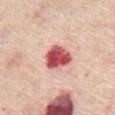Cropped from a whole-body photographic skin survey; the tile spans about 15 mm.
On the front of the torso.
A male patient approximately 75 years of age.
The lesion-visualizer software estimated an area of roughly 9.5 mm² and an outline eccentricity of about 0.25 (0 = round, 1 = elongated). It also reported an average lesion color of about L≈56 a*≈36 b*≈26 (CIELAB), roughly 21 lightness units darker than nearby skin, and a normalized lesion–skin contrast near 12.5. And it measured a border-irregularity index near 1.5/10, a color-variation rating of about 6/10, and peripheral color asymmetry of about 2. The software also gave a classifier nevus-likeness of about 0/100 and lesion-presence confidence of about 100/100.
The lesion's longest dimension is about 3.5 mm.
This is a white-light tile.